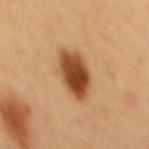A close-up tile cropped from a whole-body skin photograph, about 15 mm across. The lesion's longest dimension is about 5.5 mm. The total-body-photography lesion software estimated a footprint of about 14 mm², an outline eccentricity of about 0.8 (0 = round, 1 = elongated), and a symmetry-axis asymmetry near 0.15. The analysis additionally found a lesion color around L≈48 a*≈24 b*≈38 in CIELAB, roughly 18 lightness units darker than nearby skin, and a normalized border contrast of about 12. Located on the back. A male patient, aged around 50.